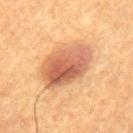{"biopsy_status": "not biopsied; imaged during a skin examination", "site": "mid back", "patient": {"sex": "male", "age_approx": 55}, "image": {"source": "total-body photography crop", "field_of_view_mm": 15}}